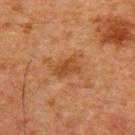follow-up: total-body-photography surveillance lesion; no biopsy | lesion diameter: ≈4 mm | illumination: cross-polarized illumination | body site: the front of the torso | acquisition: ~15 mm tile from a whole-body skin photo | patient: male, aged 58 to 62.